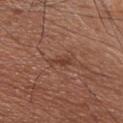Impression: The lesion was photographed on a routine skin check and not biopsied; there is no pathology result. Context: About 3 mm across. A 15 mm close-up tile from a total-body photography series done for melanoma screening. A male subject, aged around 45. Located on the chest. This is a white-light tile.